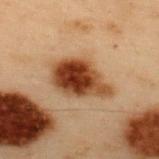Assessment: This lesion was catalogued during total-body skin photography and was not selected for biopsy. Image and clinical context: Approximately 6.5 mm at its widest. From the upper back. A male subject, in their mid- to late 50s. A close-up tile cropped from a whole-body skin photograph, about 15 mm across.